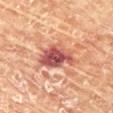follow-up — total-body-photography surveillance lesion; no biopsy | lighting — cross-polarized | image source — ~15 mm crop, total-body skin-cancer survey | anatomic site — the chest | patient — female, about 80 years old | TBP lesion metrics — an area of roughly 12 mm², an outline eccentricity of about 0.8 (0 = round, 1 = elongated), and a symmetry-axis asymmetry near 0.3; a lesion color around L≈49 a*≈28 b*≈25 in CIELAB, a lesion–skin lightness drop of about 15, and a normalized lesion–skin contrast near 11.